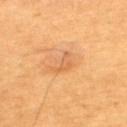Impression: This lesion was catalogued during total-body skin photography and was not selected for biopsy. Context: A close-up tile cropped from a whole-body skin photograph, about 15 mm across. Approximately 3.5 mm at its widest. Automated tile analysis of the lesion measured a footprint of about 3.5 mm² and an eccentricity of roughly 0.9. And it measured a lesion color around L≈61 a*≈25 b*≈42 in CIELAB, a lesion–skin lightness drop of about 7, and a normalized lesion–skin contrast near 4.5. The software also gave a border-irregularity index near 6.5/10, a color-variation rating of about 1.5/10, and radial color variation of about 0.5. And it measured a classifier nevus-likeness of about 15/100 and a lesion-detection confidence of about 100/100. Captured under cross-polarized illumination. The lesion is on the upper back. A male patient, aged 58–62.